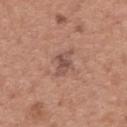Q: Was a biopsy performed?
A: imaged on a skin check; not biopsied
Q: What is the imaging modality?
A: ~15 mm tile from a whole-body skin photo
Q: How large is the lesion?
A: ~3 mm (longest diameter)
Q: What is the anatomic site?
A: the upper back
Q: What did automated image analysis measure?
A: an average lesion color of about L≈51 a*≈20 b*≈25 (CIELAB) and about 9 CIELAB-L* units darker than the surrounding skin; a border-irregularity index near 5.5/10, internal color variation of about 2.5 on a 0–10 scale, and radial color variation of about 1; lesion-presence confidence of about 100/100
Q: Patient demographics?
A: female, aged 58–62